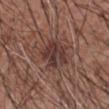This lesion was catalogued during total-body skin photography and was not selected for biopsy. A 15 mm close-up tile from a total-body photography series done for melanoma screening. A male patient, aged 58–62. Automated image analysis of the tile measured a footprint of about 11 mm², an outline eccentricity of about 0.55 (0 = round, 1 = elongated), and two-axis asymmetry of about 0.2. The software also gave a mean CIELAB color near L≈36 a*≈19 b*≈21 and about 9 CIELAB-L* units darker than the surrounding skin. The analysis additionally found border irregularity of about 2.5 on a 0–10 scale and peripheral color asymmetry of about 1.5. The software also gave an automated nevus-likeness rating near 0 out of 100 and a lesion-detection confidence of about 100/100. The lesion is on the right forearm. This is a white-light tile. Approximately 4 mm at its widest.